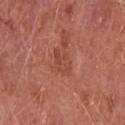Impression: Recorded during total-body skin imaging; not selected for excision or biopsy. Clinical summary: The tile uses white-light illumination. The lesion is on the chest. Approximately 3 mm at its widest. Cropped from a whole-body photographic skin survey; the tile spans about 15 mm. A male subject, approximately 65 years of age.